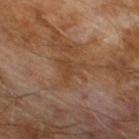  biopsy_status: not biopsied; imaged during a skin examination
  patient:
    sex: male
    age_approx: 65
  lighting: cross-polarized
  image:
    source: total-body photography crop
    field_of_view_mm: 15
  lesion_size:
    long_diameter_mm_approx: 3.5